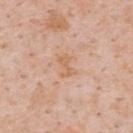{
  "biopsy_status": "not biopsied; imaged during a skin examination",
  "lesion_size": {
    "long_diameter_mm_approx": 2.5
  },
  "site": "upper back",
  "lighting": "white-light",
  "automated_metrics": {
    "area_mm2_approx": 3.5,
    "shape_asymmetry": 0.3,
    "border_irregularity_0_10": 4.5,
    "color_variation_0_10": 0.5,
    "peripheral_color_asymmetry": 0.0,
    "nevus_likeness_0_100": 0,
    "lesion_detection_confidence_0_100": 100
  },
  "image": {
    "source": "total-body photography crop",
    "field_of_view_mm": 15
  },
  "patient": {
    "sex": "male",
    "age_approx": 60
  }
}